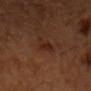Captured during whole-body skin photography for melanoma surveillance; the lesion was not biopsied. Cropped from a whole-body photographic skin survey; the tile spans about 15 mm. The lesion is located on the chest. A male patient, in their mid-40s.